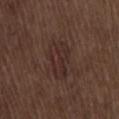  biopsy_status: not biopsied; imaged during a skin examination
  patient:
    sex: male
    age_approx: 70
  site: lower back
  lighting: white-light
  image:
    source: total-body photography crop
    field_of_view_mm: 15
  automated_metrics:
    area_mm2_approx: 11.0
    eccentricity: 0.85
    shape_asymmetry: 0.3
    border_irregularity_0_10: 3.5
    color_variation_0_10: 3.5
    peripheral_color_asymmetry: 1.0
    lesion_detection_confidence_0_100: 100
  lesion_size:
    long_diameter_mm_approx: 4.5